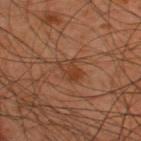Captured during whole-body skin photography for melanoma surveillance; the lesion was not biopsied.
The lesion is located on the upper back.
A region of skin cropped from a whole-body photographic capture, roughly 15 mm wide.
A male subject, approximately 45 years of age.